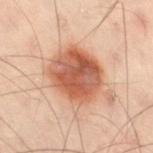Findings:
– follow-up · no biopsy performed (imaged during a skin exam)
– patient · male, aged 48–52
– site · the left leg
– imaging modality · 15 mm crop, total-body photography
– diameter · about 5.5 mm